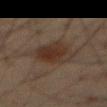workup: imaged on a skin check; not biopsied
acquisition: ~15 mm crop, total-body skin-cancer survey
lighting: cross-polarized
image-analysis metrics: an outline eccentricity of about 0.8 (0 = round, 1 = elongated) and a shape-asymmetry score of about 0.2 (0 = symmetric); an average lesion color of about L≈26 a*≈13 b*≈20 (CIELAB) and a normalized border contrast of about 7.5; border irregularity of about 2.5 on a 0–10 scale, internal color variation of about 4 on a 0–10 scale, and peripheral color asymmetry of about 1; a classifier nevus-likeness of about 75/100 and lesion-presence confidence of about 100/100
site: the abdomen
lesion diameter: ≈5.5 mm
subject: male, aged 58 to 62